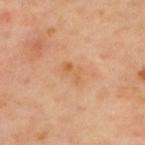Impression: The lesion was tiled from a total-body skin photograph and was not biopsied. Background: A lesion tile, about 15 mm wide, cut from a 3D total-body photograph. Imaged with cross-polarized lighting. A male subject, approximately 65 years of age. On the upper back.